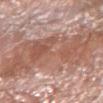{"biopsy_status": "not biopsied; imaged during a skin examination", "patient": {"sex": "male", "age_approx": 75}, "image": {"source": "total-body photography crop", "field_of_view_mm": 15}, "site": "right forearm", "lighting": "white-light", "lesion_size": {"long_diameter_mm_approx": 12.0}, "automated_metrics": {"area_mm2_approx": 60.0, "eccentricity": 0.9, "shape_asymmetry": 0.25, "border_irregularity_0_10": 6.5, "color_variation_0_10": 5.0, "peripheral_color_asymmetry": 2.0}}